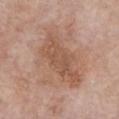Case summary:
- biopsy status · total-body-photography surveillance lesion; no biopsy
- subject · female, aged 83 or over
- lesion size · ≈8 mm
- anatomic site · the chest
- acquisition · ~15 mm tile from a whole-body skin photo
- image-analysis metrics · a lesion area of about 37 mm², an eccentricity of roughly 0.65, and two-axis asymmetry of about 0.4; a mean CIELAB color near L≈56 a*≈19 b*≈29, a lesion–skin lightness drop of about 8, and a normalized lesion–skin contrast near 6; a border-irregularity rating of about 5.5/10, internal color variation of about 5 on a 0–10 scale, and radial color variation of about 1.5